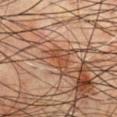Impression: Part of a total-body skin-imaging series; this lesion was reviewed on a skin check and was not flagged for biopsy. Context: The lesion is located on the chest. This is a cross-polarized tile. The patient is a male approximately 65 years of age. Automated tile analysis of the lesion measured a lesion area of about 3 mm², an outline eccentricity of about 0.9 (0 = round, 1 = elongated), and two-axis asymmetry of about 0.3. It also reported an average lesion color of about L≈37 a*≈20 b*≈28 (CIELAB) and a lesion–skin lightness drop of about 7. It also reported a border-irregularity rating of about 4/10, internal color variation of about 0 on a 0–10 scale, and a peripheral color-asymmetry measure near 0. A 15 mm crop from a total-body photograph taken for skin-cancer surveillance. Measured at roughly 3 mm in maximum diameter.